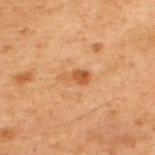Q: Was this lesion biopsied?
A: catalogued during a skin exam; not biopsied
Q: Who is the patient?
A: male, aged approximately 70
Q: What kind of image is this?
A: total-body-photography crop, ~15 mm field of view
Q: Where on the body is the lesion?
A: the upper back